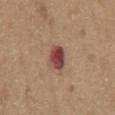Assessment:
The lesion was photographed on a routine skin check and not biopsied; there is no pathology result.
Context:
A male subject, aged 63 to 67. A region of skin cropped from a whole-body photographic capture, roughly 15 mm wide. The total-body-photography lesion software estimated a lesion area of about 6 mm², a shape eccentricity near 0.75, and two-axis asymmetry of about 0.15. And it measured a border-irregularity index near 1/10, a within-lesion color-variation index near 5.5/10, and peripheral color asymmetry of about 2. And it measured an automated nevus-likeness rating near 5 out of 100. The tile uses white-light illumination. On the chest.